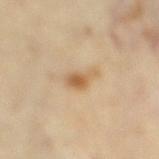{
  "biopsy_status": "not biopsied; imaged during a skin examination",
  "patient": {
    "sex": "female",
    "age_approx": 35
  },
  "automated_metrics": {
    "nevus_likeness_0_100": 80
  },
  "site": "leg",
  "image": {
    "source": "total-body photography crop",
    "field_of_view_mm": 15
  },
  "lesion_size": {
    "long_diameter_mm_approx": 3.5
  },
  "lighting": "cross-polarized"
}